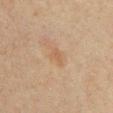  biopsy_status: not biopsied; imaged during a skin examination
  lesion_size:
    long_diameter_mm_approx: 2.5
  site: chest
  patient:
    sex: female
    age_approx: 40
  image:
    source: total-body photography crop
    field_of_view_mm: 15
  lighting: cross-polarized